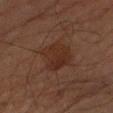follow-up: catalogued during a skin exam; not biopsied
image source: 15 mm crop, total-body photography
subject: male, aged 63–67
location: the arm
size: about 4 mm